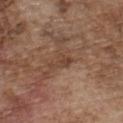The lesion was photographed on a routine skin check and not biopsied; there is no pathology result. Cropped from a total-body skin-imaging series; the visible field is about 15 mm. The tile uses white-light illumination. A male patient aged around 75. Located on the chest.